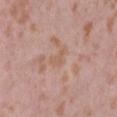Part of a total-body skin-imaging series; this lesion was reviewed on a skin check and was not flagged for biopsy.
A female patient, about 40 years old.
From the abdomen.
Automated tile analysis of the lesion measured an outline eccentricity of about 0.8 (0 = round, 1 = elongated) and a symmetry-axis asymmetry near 0.6. The software also gave a mean CIELAB color near L≈59 a*≈19 b*≈28, roughly 6 lightness units darker than nearby skin, and a lesion-to-skin contrast of about 5 (normalized; higher = more distinct). And it measured a border-irregularity index near 5.5/10, internal color variation of about 0 on a 0–10 scale, and radial color variation of about 0.
The tile uses white-light illumination.
A 15 mm close-up extracted from a 3D total-body photography capture.